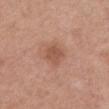Clinical impression:
The lesion was photographed on a routine skin check and not biopsied; there is no pathology result.
Acquisition and patient details:
From the mid back. Automated image analysis of the tile measured a lesion area of about 6 mm², a shape eccentricity near 0.65, and two-axis asymmetry of about 0.15. It also reported an average lesion color of about L≈53 a*≈23 b*≈30 (CIELAB) and a normalized border contrast of about 6. And it measured lesion-presence confidence of about 100/100. Cropped from a total-body skin-imaging series; the visible field is about 15 mm. This is a white-light tile. Approximately 3 mm at its widest. A female patient aged 38–42.